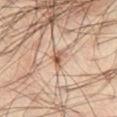Q: Was a biopsy performed?
A: no biopsy performed (imaged during a skin exam)
Q: What are the patient's age and sex?
A: male, approximately 65 years of age
Q: How was this image acquired?
A: ~15 mm tile from a whole-body skin photo
Q: Automated lesion metrics?
A: a border-irregularity rating of about 3/10 and internal color variation of about 3 on a 0–10 scale; an automated nevus-likeness rating near 90 out of 100 and lesion-presence confidence of about 100/100
Q: How was the tile lit?
A: cross-polarized illumination
Q: What is the lesion's diameter?
A: ≈2 mm
Q: What is the anatomic site?
A: the left thigh